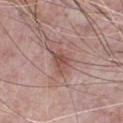No biopsy was performed on this lesion — it was imaged during a full skin examination and was not determined to be concerning. A close-up tile cropped from a whole-body skin photograph, about 15 mm across. The tile uses white-light illumination. An algorithmic analysis of the crop reported a lesion area of about 4.5 mm², a shape eccentricity near 0.75, and a shape-asymmetry score of about 0.3 (0 = symmetric). The software also gave a mean CIELAB color near L≈50 a*≈22 b*≈24, about 9 CIELAB-L* units darker than the surrounding skin, and a lesion-to-skin contrast of about 7 (normalized; higher = more distinct). The software also gave a border-irregularity rating of about 3.5/10 and radial color variation of about 1.5. Approximately 3 mm at its widest. On the chest. A male patient, aged approximately 70.